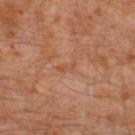| feature | finding |
|---|---|
| notes | imaged on a skin check; not biopsied |
| patient | male, approximately 30 years of age |
| site | the left leg |
| imaging modality | 15 mm crop, total-body photography |